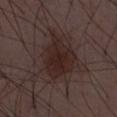Findings:
• follow-up · imaged on a skin check; not biopsied
• imaging modality · 15 mm crop, total-body photography
• subject · male, aged 48 to 52
• lighting · white-light